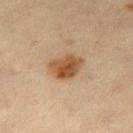Assessment:
Part of a total-body skin-imaging series; this lesion was reviewed on a skin check and was not flagged for biopsy.
Background:
The lesion is on the right thigh. A roughly 15 mm field-of-view crop from a total-body skin photograph. Automated tile analysis of the lesion measured a footprint of about 8.5 mm² and a shape-asymmetry score of about 0.25 (0 = symmetric). It also reported an automated nevus-likeness rating near 100 out of 100 and a detector confidence of about 100 out of 100 that the crop contains a lesion. Imaged with cross-polarized lighting. A female subject approximately 45 years of age. Approximately 4 mm at its widest.